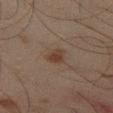No biopsy was performed on this lesion — it was imaged during a full skin examination and was not determined to be concerning.
A 15 mm close-up tile from a total-body photography series done for melanoma screening.
Measured at roughly 2.5 mm in maximum diameter.
Located on the left thigh.
The tile uses cross-polarized illumination.
A male subject, in their mid- to late 40s.